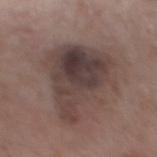The lesion was tiled from a total-body skin photograph and was not biopsied. Captured under white-light illumination. From the mid back. The subject is a male roughly 65 years of age. The total-body-photography lesion software estimated an average lesion color of about L≈40 a*≈14 b*≈18 (CIELAB) and about 11 CIELAB-L* units darker than the surrounding skin. And it measured border irregularity of about 6 on a 0–10 scale and internal color variation of about 7 on a 0–10 scale. It also reported an automated nevus-likeness rating near 0 out of 100. A 15 mm close-up extracted from a 3D total-body photography capture.